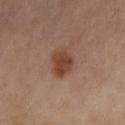Recorded during total-body skin imaging; not selected for excision or biopsy.
A female patient, aged approximately 70.
A roughly 15 mm field-of-view crop from a total-body skin photograph.
Captured under cross-polarized illumination.
About 3.5 mm across.
From the left lower leg.